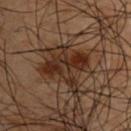The lesion was tiled from a total-body skin photograph and was not biopsied. About 5 mm across. Located on the right upper arm. Imaged with cross-polarized lighting. A male subject, in their 50s. An algorithmic analysis of the crop reported a lesion color around L≈21 a*≈14 b*≈21 in CIELAB and a normalized lesion–skin contrast near 9.5. And it measured a classifier nevus-likeness of about 10/100 and a detector confidence of about 95 out of 100 that the crop contains a lesion. Cropped from a whole-body photographic skin survey; the tile spans about 15 mm.